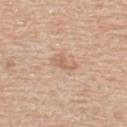{
  "biopsy_status": "not biopsied; imaged during a skin examination",
  "patient": {
    "sex": "male",
    "age_approx": 60
  },
  "site": "back",
  "automated_metrics": {
    "shape_asymmetry": 0.45,
    "cielab_L": 63,
    "cielab_a": 19,
    "cielab_b": 32,
    "vs_skin_darker_L": 7.0,
    "vs_skin_contrast_norm": 5.5,
    "border_irregularity_0_10": 5.0,
    "color_variation_0_10": 2.0,
    "nevus_likeness_0_100": 0,
    "lesion_detection_confidence_0_100": 100
  },
  "image": {
    "source": "total-body photography crop",
    "field_of_view_mm": 15
  },
  "lesion_size": {
    "long_diameter_mm_approx": 3.0
  }
}